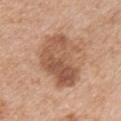Q: Was this lesion biopsied?
A: no biopsy performed (imaged during a skin exam)
Q: What did automated image analysis measure?
A: an area of roughly 24 mm², a shape eccentricity near 0.75, and a symmetry-axis asymmetry near 0.3; a lesion color around L≈55 a*≈21 b*≈32 in CIELAB and a lesion–skin lightness drop of about 11; a classifier nevus-likeness of about 40/100 and a detector confidence of about 100 out of 100 that the crop contains a lesion
Q: What are the patient's age and sex?
A: female, approximately 40 years of age
Q: Lesion location?
A: the chest
Q: How large is the lesion?
A: about 7 mm
Q: What kind of image is this?
A: 15 mm crop, total-body photography
Q: What lighting was used for the tile?
A: white-light illumination